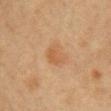{
  "biopsy_status": "not biopsied; imaged during a skin examination",
  "image": {
    "source": "total-body photography crop",
    "field_of_view_mm": 15
  },
  "site": "chest",
  "lighting": "cross-polarized",
  "lesion_size": {
    "long_diameter_mm_approx": 3.0
  },
  "patient": {
    "sex": "female",
    "age_approx": 30
  }
}